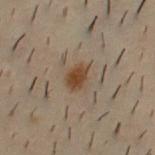Q: Was this lesion biopsied?
A: catalogued during a skin exam; not biopsied
Q: What is the anatomic site?
A: the front of the torso
Q: What did automated image analysis measure?
A: a color-variation rating of about 2.5/10 and a peripheral color-asymmetry measure near 0.5
Q: Patient demographics?
A: male, aged 28–32
Q: What kind of image is this?
A: ~15 mm tile from a whole-body skin photo